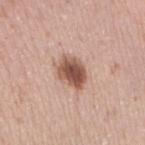Clinical impression: The lesion was photographed on a routine skin check and not biopsied; there is no pathology result. Acquisition and patient details: The total-body-photography lesion software estimated an average lesion color of about L≈53 a*≈21 b*≈27 (CIELAB) and a lesion-to-skin contrast of about 10.5 (normalized; higher = more distinct). Longest diameter approximately 3.5 mm. A female patient, about 50 years old. Cropped from a whole-body photographic skin survey; the tile spans about 15 mm. Located on the arm.